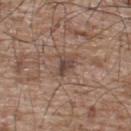biopsy status = no biopsy performed (imaged during a skin exam); image = total-body-photography crop, ~15 mm field of view; location = the upper back; lesion diameter = about 3 mm; patient = male, in their mid-60s; image-analysis metrics = a detector confidence of about 100 out of 100 that the crop contains a lesion; lighting = white-light.